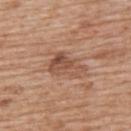Recorded during total-body skin imaging; not selected for excision or biopsy. The lesion-visualizer software estimated an area of roughly 8.5 mm², an outline eccentricity of about 0.8 (0 = round, 1 = elongated), and two-axis asymmetry of about 0.4. The analysis additionally found a border-irregularity rating of about 4.5/10, a within-lesion color-variation index near 6/10, and peripheral color asymmetry of about 2. A lesion tile, about 15 mm wide, cut from a 3D total-body photograph. The lesion is on the upper back. A male subject approximately 60 years of age.